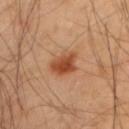• notes · total-body-photography surveillance lesion; no biopsy
• diameter · ≈3 mm
• imaging modality · total-body-photography crop, ~15 mm field of view
• subject · male, about 40 years old
• body site · the upper back
• tile lighting · cross-polarized